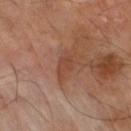Q: Who is the patient?
A: male, aged approximately 70
Q: What kind of image is this?
A: ~15 mm crop, total-body skin-cancer survey
Q: Automated lesion metrics?
A: a footprint of about 4.5 mm² and an eccentricity of roughly 0.85; a lesion color around L≈43 a*≈22 b*≈29 in CIELAB, a lesion–skin lightness drop of about 7, and a lesion-to-skin contrast of about 5.5 (normalized; higher = more distinct); lesion-presence confidence of about 100/100
Q: Lesion location?
A: the right thigh
Q: How large is the lesion?
A: about 3.5 mm